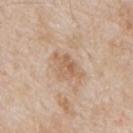Case summary:
- workup · no biopsy performed (imaged during a skin exam)
- anatomic site · the mid back
- automated metrics · a nevus-likeness score of about 0/100 and lesion-presence confidence of about 100/100
- subject · male, approximately 65 years of age
- tile lighting · white-light
- imaging modality · ~15 mm crop, total-body skin-cancer survey